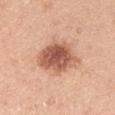| feature | finding |
|---|---|
| follow-up | no biopsy performed (imaged during a skin exam) |
| location | the right upper arm |
| patient | male, aged around 45 |
| image | total-body-photography crop, ~15 mm field of view |
| lesion diameter | ~5.5 mm (longest diameter) |
| lighting | white-light |